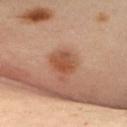Located on the front of the torso. An algorithmic analysis of the crop reported an area of roughly 7.5 mm² and a symmetry-axis asymmetry near 0.3. The software also gave a lesion color around L≈50 a*≈24 b*≈33 in CIELAB, about 9 CIELAB-L* units darker than the surrounding skin, and a normalized border contrast of about 7. And it measured a classifier nevus-likeness of about 60/100 and a detector confidence of about 100 out of 100 that the crop contains a lesion. Longest diameter approximately 3.5 mm. A female patient, roughly 45 years of age. Imaged with cross-polarized lighting. A roughly 15 mm field-of-view crop from a total-body skin photograph.